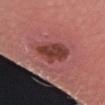{"site": "arm", "patient": {"sex": "male", "age_approx": 35}, "image": {"source": "total-body photography crop", "field_of_view_mm": 15}, "lighting": "white-light", "lesion_size": {"long_diameter_mm_approx": 5.0}, "automated_metrics": {"cielab_L": 41, "cielab_a": 27, "cielab_b": 25, "vs_skin_contrast_norm": 9.0, "border_irregularity_0_10": 2.5, "color_variation_0_10": 4.0, "peripheral_color_asymmetry": 1.0}}